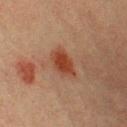imaging modality: total-body-photography crop, ~15 mm field of view
anatomic site: the front of the torso
diameter: ≈4 mm
subject: male, about 40 years old
image-analysis metrics: an area of roughly 6.5 mm² and two-axis asymmetry of about 0.2; a within-lesion color-variation index near 2.5/10 and radial color variation of about 0.5
lighting: cross-polarized illumination